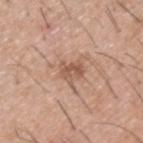Clinical summary:
Located on the upper back. This is a white-light tile. A lesion tile, about 15 mm wide, cut from a 3D total-body photograph. Approximately 2.5 mm at its widest. A male subject aged around 40.